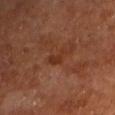Assessment:
No biopsy was performed on this lesion — it was imaged during a full skin examination and was not determined to be concerning.
Acquisition and patient details:
Approximately 3 mm at its widest. A male subject, aged approximately 60. The lesion-visualizer software estimated a mean CIELAB color near L≈31 a*≈22 b*≈29 and a lesion-to-skin contrast of about 6 (normalized; higher = more distinct). And it measured border irregularity of about 5 on a 0–10 scale, a color-variation rating of about 1.5/10, and peripheral color asymmetry of about 0.5. Cropped from a whole-body photographic skin survey; the tile spans about 15 mm. Captured under cross-polarized illumination. The lesion is located on the left upper arm.